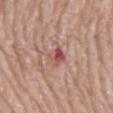This lesion was catalogued during total-body skin photography and was not selected for biopsy.
This is a white-light tile.
A male subject, aged 73–77.
Measured at roughly 3 mm in maximum diameter.
Cropped from a total-body skin-imaging series; the visible field is about 15 mm.
The lesion is located on the mid back.